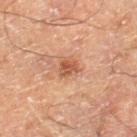Impression:
No biopsy was performed on this lesion — it was imaged during a full skin examination and was not determined to be concerning.
Image and clinical context:
A lesion tile, about 15 mm wide, cut from a 3D total-body photograph. From the leg. A male subject about 60 years old.